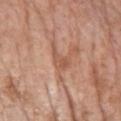Recorded during total-body skin imaging; not selected for excision or biopsy.
Approximately 3 mm at its widest.
A lesion tile, about 15 mm wide, cut from a 3D total-body photograph.
The lesion is on the chest.
The patient is a female aged 68–72.
Captured under white-light illumination.
Automated tile analysis of the lesion measured a lesion area of about 4 mm², an eccentricity of roughly 0.75, and a shape-asymmetry score of about 0.45 (0 = symmetric). And it measured a mean CIELAB color near L≈55 a*≈23 b*≈31 and a normalized lesion–skin contrast near 5.5. It also reported a border-irregularity rating of about 4.5/10, a within-lesion color-variation index near 2.5/10, and a peripheral color-asymmetry measure near 0.5.